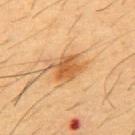From the chest.
A male subject about 55 years old.
A close-up tile cropped from a whole-body skin photograph, about 15 mm across.
Imaged with cross-polarized lighting.